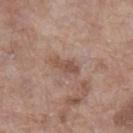The lesion was tiled from a total-body skin photograph and was not biopsied.
The patient is a female aged approximately 85 or older.
Located on the left lower leg.
Approximately 3.5 mm at its widest.
The tile uses white-light illumination.
A close-up tile cropped from a whole-body skin photograph, about 15 mm across.